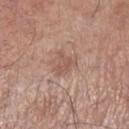{
  "biopsy_status": "not biopsied; imaged during a skin examination",
  "lighting": "white-light",
  "automated_metrics": {
    "area_mm2_approx": 4.0,
    "eccentricity": 0.55,
    "shape_asymmetry": 0.45,
    "cielab_L": 54,
    "cielab_a": 20,
    "cielab_b": 25,
    "vs_skin_darker_L": 8.0,
    "vs_skin_contrast_norm": 5.5,
    "nevus_likeness_0_100": 0,
    "lesion_detection_confidence_0_100": 100
  },
  "patient": {
    "sex": "male",
    "age_approx": 70
  },
  "image": {
    "source": "total-body photography crop",
    "field_of_view_mm": 15
  },
  "site": "left lower leg"
}